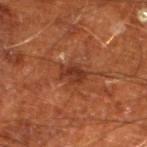{
  "biopsy_status": "not biopsied; imaged during a skin examination",
  "lesion_size": {
    "long_diameter_mm_approx": 3.0
  },
  "site": "left lower leg",
  "lighting": "cross-polarized",
  "image": {
    "source": "total-body photography crop",
    "field_of_view_mm": 15
  },
  "patient": {
    "sex": "male",
    "age_approx": 65
  }
}